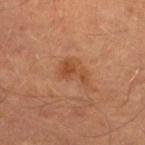<record>
<biopsy_status>not biopsied; imaged during a skin examination</biopsy_status>
<site>left lower leg</site>
<lesion_size>
  <long_diameter_mm_approx>3.5</long_diameter_mm_approx>
</lesion_size>
<image>
  <source>total-body photography crop</source>
  <field_of_view_mm>15</field_of_view_mm>
</image>
<automated_metrics>
  <area_mm2_approx>5.0</area_mm2_approx>
  <eccentricity>0.85</eccentricity>
  <shape_asymmetry>0.55</shape_asymmetry>
  <nevus_likeness_0_100>0</nevus_likeness_0_100>
  <lesion_detection_confidence_0_100>100</lesion_detection_confidence_0_100>
</automated_metrics>
<patient>
  <sex>male</sex>
  <age_approx>40</age_approx>
</patient>
<lighting>cross-polarized</lighting>
</record>